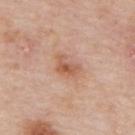Q: Is there a histopathology result?
A: no biopsy performed (imaged during a skin exam)
Q: What kind of image is this?
A: 15 mm crop, total-body photography
Q: Illumination type?
A: white-light
Q: Where on the body is the lesion?
A: the upper back
Q: Patient demographics?
A: male, about 75 years old
Q: Lesion size?
A: ~3 mm (longest diameter)